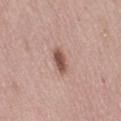Clinical summary:
A close-up tile cropped from a whole-body skin photograph, about 15 mm across. A female subject about 50 years old. The lesion-visualizer software estimated a lesion area of about 5 mm² and an outline eccentricity of about 0.9 (0 = round, 1 = elongated). The analysis additionally found a within-lesion color-variation index near 2.5/10. Longest diameter approximately 3.5 mm. The lesion is located on the abdomen.